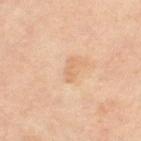Findings:
• notes · no biopsy performed (imaged during a skin exam)
• diameter · about 3 mm
• subject · female, roughly 50 years of age
• automated metrics · a border-irregularity index near 6/10 and a peripheral color-asymmetry measure near 0
• site · the right thigh
• acquisition · ~15 mm tile from a whole-body skin photo